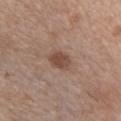Impression: Imaged during a routine full-body skin examination; the lesion was not biopsied and no histopathology is available. Image and clinical context: A region of skin cropped from a whole-body photographic capture, roughly 15 mm wide. The lesion-visualizer software estimated a border-irregularity rating of about 1.5/10. The software also gave lesion-presence confidence of about 100/100. This is a white-light tile. A female subject approximately 40 years of age. The lesion is located on the front of the torso.